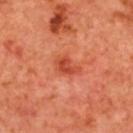| field | value |
|---|---|
| image | 15 mm crop, total-body photography |
| automated lesion analysis | a shape eccentricity near 0.75; an average lesion color of about L≈51 a*≈38 b*≈39 (CIELAB), roughly 10 lightness units darker than nearby skin, and a lesion-to-skin contrast of about 7 (normalized; higher = more distinct); a border-irregularity rating of about 3.5/10 and peripheral color asymmetry of about 1; an automated nevus-likeness rating near 30 out of 100 and lesion-presence confidence of about 100/100 |
| subject | female, roughly 40 years of age |
| lesion diameter | ~3 mm (longest diameter) |
| tile lighting | cross-polarized |
| body site | the upper back |